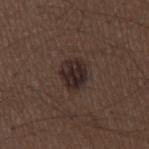Impression:
No biopsy was performed on this lesion — it was imaged during a full skin examination and was not determined to be concerning.
Image and clinical context:
The subject is a male aged around 50. From the back. A 15 mm crop from a total-body photograph taken for skin-cancer surveillance. Approximately 3.5 mm at its widest.